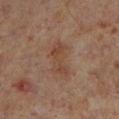No biopsy was performed on this lesion — it was imaged during a full skin examination and was not determined to be concerning. This image is a 15 mm lesion crop taken from a total-body photograph. Captured under cross-polarized illumination. The patient is a male aged around 60. Measured at roughly 4.5 mm in maximum diameter. From the left lower leg.